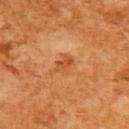{
  "biopsy_status": "not biopsied; imaged during a skin examination",
  "automated_metrics": {
    "area_mm2_approx": 4.0,
    "eccentricity": 0.7,
    "shape_asymmetry": 0.2,
    "color_variation_0_10": 3.0,
    "peripheral_color_asymmetry": 1.0,
    "nevus_likeness_0_100": 15
  },
  "lesion_size": {
    "long_diameter_mm_approx": 2.5
  },
  "patient": {
    "sex": "female",
    "age_approx": 55
  },
  "image": {
    "source": "total-body photography crop",
    "field_of_view_mm": 15
  },
  "site": "upper back"
}